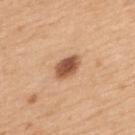Notes:
– workup: total-body-photography surveillance lesion; no biopsy
– patient: female, approximately 30 years of age
– anatomic site: the upper back
– automated lesion analysis: an area of roughly 6 mm² and a shape eccentricity near 0.8; a within-lesion color-variation index near 4/10; a classifier nevus-likeness of about 100/100 and lesion-presence confidence of about 100/100
– lesion size: about 3.5 mm
– tile lighting: white-light illumination
– image source: total-body-photography crop, ~15 mm field of view